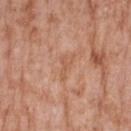The lesion was photographed on a routine skin check and not biopsied; there is no pathology result. A female patient, aged around 75. From the right forearm. Cropped from a total-body skin-imaging series; the visible field is about 15 mm. The tile uses white-light illumination. An algorithmic analysis of the crop reported an area of roughly 3 mm², a shape eccentricity near 0.9, and a symmetry-axis asymmetry near 0.55. The analysis additionally found a lesion color around L≈57 a*≈23 b*≈33 in CIELAB, roughly 6 lightness units darker than nearby skin, and a normalized lesion–skin contrast near 4.5. The analysis additionally found a classifier nevus-likeness of about 0/100 and lesion-presence confidence of about 100/100. Measured at roughly 3 mm in maximum diameter.